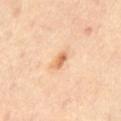Recorded during total-body skin imaging; not selected for excision or biopsy.
A region of skin cropped from a whole-body photographic capture, roughly 15 mm wide.
The tile uses cross-polarized illumination.
The lesion is on the abdomen.
The recorded lesion diameter is about 2.5 mm.
A male patient about 65 years old.